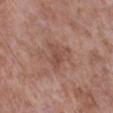A male patient aged approximately 55.
On the right lower leg.
A roughly 15 mm field-of-view crop from a total-body skin photograph.